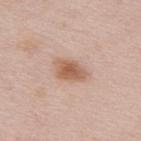Recorded during total-body skin imaging; not selected for excision or biopsy.
A close-up tile cropped from a whole-body skin photograph, about 15 mm across.
The lesion is located on the upper back.
Approximately 4 mm at its widest.
The patient is a female in their mid- to late 60s.